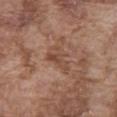The lesion was photographed on a routine skin check and not biopsied; there is no pathology result.
The lesion is on the abdomen.
A 15 mm close-up tile from a total-body photography series done for melanoma screening.
Approximately 4 mm at its widest.
This is a white-light tile.
A male subject, approximately 75 years of age.
Automated image analysis of the tile measured border irregularity of about 5 on a 0–10 scale and radial color variation of about 1.5.